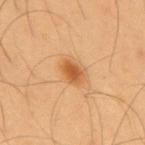biopsy status: total-body-photography surveillance lesion; no biopsy | anatomic site: the upper back | image source: total-body-photography crop, ~15 mm field of view | subject: male, aged 53 to 57.